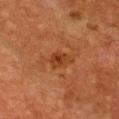Q: Was this lesion biopsied?
A: total-body-photography surveillance lesion; no biopsy
Q: What is the anatomic site?
A: the chest
Q: How was this image acquired?
A: ~15 mm crop, total-body skin-cancer survey
Q: Lesion size?
A: ~2.5 mm (longest diameter)
Q: What are the patient's age and sex?
A: female, aged around 50
Q: Automated lesion metrics?
A: a footprint of about 4.5 mm², a shape eccentricity near 0.6, and two-axis asymmetry of about 0.25; a normalized lesion–skin contrast near 7; a border-irregularity rating of about 2/10 and a color-variation rating of about 3.5/10; an automated nevus-likeness rating near 30 out of 100 and lesion-presence confidence of about 100/100